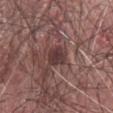image:
  source: total-body photography crop
  field_of_view_mm: 15
site: arm
automated_metrics:
  color_variation_0_10: 3.0
  peripheral_color_asymmetry: 1.0
  nevus_likeness_0_100: 0
lesion_size:
  long_diameter_mm_approx: 2.5
patient:
  sex: male
  age_approx: 60
lighting: white-light